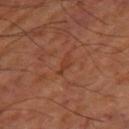This lesion was catalogued during total-body skin photography and was not selected for biopsy. Automated tile analysis of the lesion measured a lesion color around L≈38 a*≈25 b*≈31 in CIELAB, roughly 5 lightness units darker than nearby skin, and a normalized lesion–skin contrast near 5. The analysis additionally found a color-variation rating of about 0/10 and radial color variation of about 0. Imaged with cross-polarized lighting. Located on the right thigh. A subject aged 63 to 67. A roughly 15 mm field-of-view crop from a total-body skin photograph.